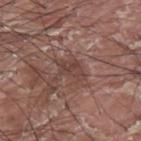Q: Is there a histopathology result?
A: catalogued during a skin exam; not biopsied
Q: What kind of image is this?
A: ~15 mm tile from a whole-body skin photo
Q: What are the patient's age and sex?
A: male, roughly 40 years of age
Q: What is the anatomic site?
A: the upper back
Q: What is the lesion's diameter?
A: ≈3.5 mm
Q: Illumination type?
A: white-light illumination